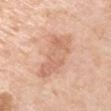Assessment:
No biopsy was performed on this lesion — it was imaged during a full skin examination and was not determined to be concerning.
Acquisition and patient details:
A male patient about 60 years old. This image is a 15 mm lesion crop taken from a total-body photograph. Automated tile analysis of the lesion measured a footprint of about 16 mm². The analysis additionally found a mean CIELAB color near L≈66 a*≈22 b*≈32 and a normalized lesion–skin contrast near 5.5. Measured at roughly 6.5 mm in maximum diameter. The lesion is on the left upper arm.